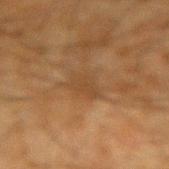Case summary:
• illumination: cross-polarized illumination
• size: about 4 mm
• subject: male, in their mid- to late 60s
• imaging modality: total-body-photography crop, ~15 mm field of view
• TBP lesion metrics: a nevus-likeness score of about 0/100
• anatomic site: the arm